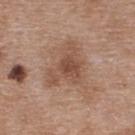The lesion was photographed on a routine skin check and not biopsied; there is no pathology result. The subject is a female aged 38 to 42. A roughly 15 mm field-of-view crop from a total-body skin photograph. Located on the upper back. Captured under white-light illumination. The total-body-photography lesion software estimated a border-irregularity index near 6/10 and a within-lesion color-variation index near 4/10. And it measured a classifier nevus-likeness of about 10/100 and lesion-presence confidence of about 100/100. Longest diameter approximately 6 mm.